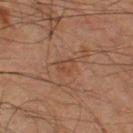workup = total-body-photography surveillance lesion; no biopsy
location = the right thigh
illumination = cross-polarized illumination
lesion diameter = about 2.5 mm
image = ~15 mm tile from a whole-body skin photo
subject = male, approximately 60 years of age